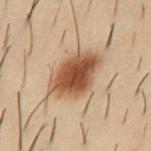follow-up — imaged on a skin check; not biopsied
imaging modality — 15 mm crop, total-body photography
subject — male, aged around 30
site — the abdomen